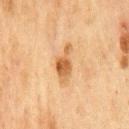This lesion was catalogued during total-body skin photography and was not selected for biopsy.
The lesion is on the chest.
A 15 mm close-up extracted from a 3D total-body photography capture.
Captured under cross-polarized illumination.
Measured at roughly 4.5 mm in maximum diameter.
The subject is a male approximately 75 years of age.